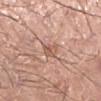biopsy status: total-body-photography surveillance lesion; no biopsy
lighting: white-light
diameter: about 2.5 mm
location: the left lower leg
imaging modality: total-body-photography crop, ~15 mm field of view
subject: male, in their 20s